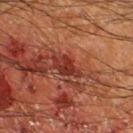No biopsy was performed on this lesion — it was imaged during a full skin examination and was not determined to be concerning. The lesion-visualizer software estimated an area of roughly 5 mm². The analysis additionally found a detector confidence of about 90 out of 100 that the crop contains a lesion. A region of skin cropped from a whole-body photographic capture, roughly 15 mm wide. Imaged with cross-polarized lighting. The lesion is on the left lower leg. The patient is a male aged around 65. About 3.5 mm across.